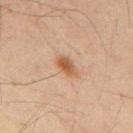<lesion>
<patient>
  <sex>male</sex>
  <age_approx>50</age_approx>
</patient>
<site>back</site>
<image>
  <source>total-body photography crop</source>
  <field_of_view_mm>15</field_of_view_mm>
</image>
<lesion_size>
  <long_diameter_mm_approx>3.0</long_diameter_mm_approx>
</lesion_size>
</lesion>